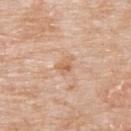workup = total-body-photography surveillance lesion; no biopsy
diameter = ~2.5 mm (longest diameter)
subject = male, aged 73 to 77
lighting = white-light illumination
location = the upper back
image = total-body-photography crop, ~15 mm field of view
automated lesion analysis = an average lesion color of about L≈64 a*≈20 b*≈34 (CIELAB) and roughly 8 lightness units darker than nearby skin; a border-irregularity index near 3.5/10, a within-lesion color-variation index near 3.5/10, and radial color variation of about 1.5; lesion-presence confidence of about 100/100